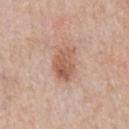Imaged during a routine full-body skin examination; the lesion was not biopsied and no histopathology is available. Automated image analysis of the tile measured a lesion color around L≈58 a*≈21 b*≈30 in CIELAB, a lesion–skin lightness drop of about 10, and a normalized border contrast of about 7.5. And it measured a border-irregularity index near 2.5/10, a color-variation rating of about 5/10, and a peripheral color-asymmetry measure near 2. It also reported a classifier nevus-likeness of about 40/100 and a detector confidence of about 100 out of 100 that the crop contains a lesion. The tile uses white-light illumination. A male patient aged 58–62. A 15 mm crop from a total-body photograph taken for skin-cancer surveillance. Located on the abdomen.